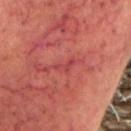This lesion was catalogued during total-body skin photography and was not selected for biopsy.
Automated image analysis of the tile measured a footprint of about 2.5 mm². The software also gave a lesion color around L≈42 a*≈34 b*≈24 in CIELAB, about 6 CIELAB-L* units darker than the surrounding skin, and a lesion-to-skin contrast of about 5 (normalized; higher = more distinct). It also reported a border-irregularity rating of about 5/10 and peripheral color asymmetry of about 0. The software also gave a classifier nevus-likeness of about 0/100 and a lesion-detection confidence of about 65/100.
A roughly 15 mm field-of-view crop from a total-body skin photograph.
Captured under cross-polarized illumination.
Measured at roughly 2.5 mm in maximum diameter.
The subject is a male aged approximately 60.
On the head or neck.